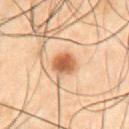This lesion was catalogued during total-body skin photography and was not selected for biopsy.
Automated tile analysis of the lesion measured an area of roughly 6.5 mm², an outline eccentricity of about 0.5 (0 = round, 1 = elongated), and a shape-asymmetry score of about 0.15 (0 = symmetric). And it measured a mean CIELAB color near L≈46 a*≈19 b*≈30, a lesion–skin lightness drop of about 12, and a normalized lesion–skin contrast near 9.5.
A roughly 15 mm field-of-view crop from a total-body skin photograph.
The lesion is on the abdomen.
The lesion's longest dimension is about 3 mm.
A male subject aged 48–52.